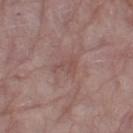  biopsy_status: not biopsied; imaged during a skin examination
  lesion_size:
    long_diameter_mm_approx: 3.0
  automated_metrics:
    shape_asymmetry: 0.55
    vs_skin_darker_L: 6.0
    vs_skin_contrast_norm: 4.5
    border_irregularity_0_10: 6.0
    peripheral_color_asymmetry: 0.5
    nevus_likeness_0_100: 0
    lesion_detection_confidence_0_100: 100
  patient:
    sex: female
    age_approx: 65
  site: leg
  image:
    source: total-body photography crop
    field_of_view_mm: 15